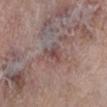follow-up = catalogued during a skin exam; not biopsied | illumination = white-light illumination | body site = the leg | subject = female, aged approximately 65 | image source = ~15 mm tile from a whole-body skin photo.